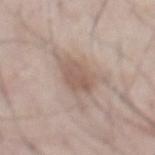| feature | finding |
|---|---|
| anatomic site | the abdomen |
| acquisition | total-body-photography crop, ~15 mm field of view |
| subject | male, roughly 75 years of age |